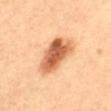This lesion was catalogued during total-body skin photography and was not selected for biopsy.
The patient is a male about 20 years old.
Located on the mid back.
About 6 mm across.
The total-body-photography lesion software estimated two-axis asymmetry of about 0.2. It also reported a mean CIELAB color near L≈59 a*≈26 b*≈38 and about 17 CIELAB-L* units darker than the surrounding skin. The software also gave a within-lesion color-variation index near 6.5/10 and a peripheral color-asymmetry measure near 2.
A 15 mm close-up extracted from a 3D total-body photography capture.
Captured under cross-polarized illumination.